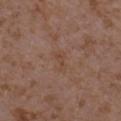Notes:
– workup: imaged on a skin check; not biopsied
– lesion size: about 2.5 mm
– illumination: white-light
– image source: 15 mm crop, total-body photography
– patient: female, in their mid-30s
– location: the left upper arm